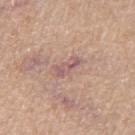<case>
<biopsy_status>not biopsied; imaged during a skin examination</biopsy_status>
<automated_metrics>
  <area_mm2_approx>5.0</area_mm2_approx>
  <eccentricity>0.9</eccentricity>
  <cielab_L>57</cielab_L>
  <cielab_a>20</cielab_a>
  <cielab_b>21</cielab_b>
  <vs_skin_darker_L>8.0</vs_skin_darker_L>
  <vs_skin_contrast_norm>6.5</vs_skin_contrast_norm>
  <border_irregularity_0_10>3.5</border_irregularity_0_10>
  <peripheral_color_asymmetry>1.0</peripheral_color_asymmetry>
  <nevus_likeness_0_100>0</nevus_likeness_0_100>
  <lesion_detection_confidence_0_100>70</lesion_detection_confidence_0_100>
</automated_metrics>
<lighting>white-light</lighting>
<patient>
  <sex>female</sex>
  <age_approx>70</age_approx>
</patient>
<image>
  <source>total-body photography crop</source>
  <field_of_view_mm>15</field_of_view_mm>
</image>
<site>leg</site>
</case>